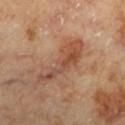{
  "biopsy_status": "not biopsied; imaged during a skin examination",
  "site": "left lower leg",
  "lesion_size": {
    "long_diameter_mm_approx": 7.5
  },
  "automated_metrics": {
    "cielab_L": 51,
    "cielab_a": 23,
    "cielab_b": 31,
    "vs_skin_darker_L": 9.0,
    "vs_skin_contrast_norm": 6.5,
    "nevus_likeness_0_100": 0,
    "lesion_detection_confidence_0_100": 100
  },
  "patient": {
    "sex": "female",
    "age_approx": 60
  },
  "image": {
    "source": "total-body photography crop",
    "field_of_view_mm": 15
  },
  "lighting": "cross-polarized"
}